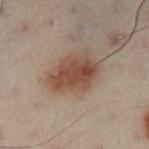<record>
<biopsy_status>not biopsied; imaged during a skin examination</biopsy_status>
<automated_metrics>
  <area_mm2_approx>11.0</area_mm2_approx>
  <eccentricity>0.85</eccentricity>
  <vs_skin_darker_L>10.0</vs_skin_darker_L>
  <vs_skin_contrast_norm>9.5</vs_skin_contrast_norm>
  <nevus_likeness_0_100>100</nevus_likeness_0_100>
  <lesion_detection_confidence_0_100>100</lesion_detection_confidence_0_100>
</automated_metrics>
<patient>
  <sex>male</sex>
  <age_approx>50</age_approx>
</patient>
<lighting>cross-polarized</lighting>
<site>left lower leg</site>
<image>
  <source>total-body photography crop</source>
  <field_of_view_mm>15</field_of_view_mm>
</image>
<lesion_size>
  <long_diameter_mm_approx>5.0</long_diameter_mm_approx>
</lesion_size>
</record>